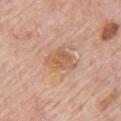Clinical impression:
No biopsy was performed on this lesion — it was imaged during a full skin examination and was not determined to be concerning.
Context:
The lesion's longest dimension is about 4 mm. The lesion is located on the chest. Captured under white-light illumination. Cropped from a whole-body photographic skin survey; the tile spans about 15 mm. The subject is a male aged 78–82.